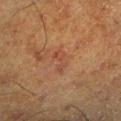Context:
On the leg. Automated image analysis of the tile measured a shape eccentricity near 0.9. The software also gave an automated nevus-likeness rating near 0 out of 100 and a lesion-detection confidence of about 100/100. A male patient about 60 years old. Cropped from a whole-body photographic skin survey; the tile spans about 15 mm.